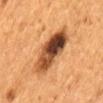The lesion was tiled from a total-body skin photograph and was not biopsied. Imaged with cross-polarized lighting. The lesion is located on the lower back. Cropped from a total-body skin-imaging series; the visible field is about 15 mm. Automated tile analysis of the lesion measured a lesion area of about 17 mm², a shape eccentricity near 0.8, and a symmetry-axis asymmetry near 0.15. It also reported a border-irregularity index near 2/10 and radial color variation of about 4.5. A female patient, aged approximately 50. The recorded lesion diameter is about 6 mm.